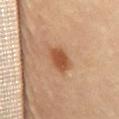Part of a total-body skin-imaging series; this lesion was reviewed on a skin check and was not flagged for biopsy.